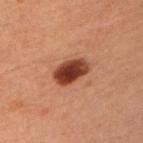{"biopsy_status": "not biopsied; imaged during a skin examination", "site": "left upper arm", "automated_metrics": {"cielab_L": 29, "cielab_a": 22, "cielab_b": 25, "vs_skin_darker_L": 16.0, "vs_skin_contrast_norm": 14.0, "border_irregularity_0_10": 2.0, "color_variation_0_10": 3.5, "peripheral_color_asymmetry": 1.0}, "lesion_size": {"long_diameter_mm_approx": 3.5}, "patient": {"sex": "male", "age_approx": 60}, "lighting": "cross-polarized", "image": {"source": "total-body photography crop", "field_of_view_mm": 15}}